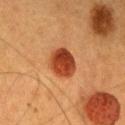<lesion>
<biopsy_status>not biopsied; imaged during a skin examination</biopsy_status>
</lesion>